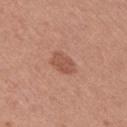Notes:
• biopsy status · no biopsy performed (imaged during a skin exam)
• patient · male, about 45 years old
• acquisition · ~15 mm crop, total-body skin-cancer survey
• site · the left upper arm